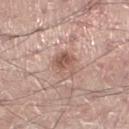biopsy status: catalogued during a skin exam; not biopsied
lesion diameter: ~3.5 mm (longest diameter)
patient: male, aged 28 to 32
automated metrics: lesion-presence confidence of about 100/100
image source: ~15 mm crop, total-body skin-cancer survey
tile lighting: white-light illumination
body site: the left lower leg